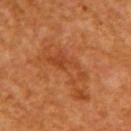Q: What is the anatomic site?
A: the right upper arm
Q: Lesion size?
A: ≈6 mm
Q: Automated lesion metrics?
A: a color-variation rating of about 5.5/10 and peripheral color asymmetry of about 2; a classifier nevus-likeness of about 0/100
Q: What kind of image is this?
A: 15 mm crop, total-body photography
Q: What lighting was used for the tile?
A: cross-polarized
Q: Patient demographics?
A: female, roughly 55 years of age